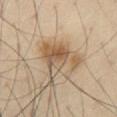Captured during whole-body skin photography for melanoma surveillance; the lesion was not biopsied.
A male subject aged 53 to 57.
On the abdomen.
About 5.5 mm across.
Cropped from a whole-body photographic skin survey; the tile spans about 15 mm.
The tile uses cross-polarized illumination.